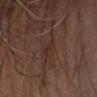notes: total-body-photography surveillance lesion; no biopsy
patient: male, about 70 years old
acquisition: ~15 mm tile from a whole-body skin photo
automated metrics: a mean CIELAB color near L≈27 a*≈17 b*≈21, roughly 4 lightness units darker than nearby skin, and a normalized lesion–skin contrast near 5; a border-irregularity index near 3/10, internal color variation of about 0 on a 0–10 scale, and peripheral color asymmetry of about 0; lesion-presence confidence of about 75/100
lesion diameter: ≈3 mm
tile lighting: cross-polarized
anatomic site: the left forearm